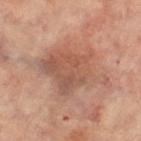  biopsy_status: not biopsied; imaged during a skin examination
  site: left leg
  patient:
    sex: female
    age_approx: 65
  image:
    source: total-body photography crop
    field_of_view_mm: 15